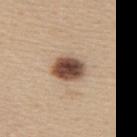Captured during whole-body skin photography for melanoma surveillance; the lesion was not biopsied. Automated image analysis of the tile measured a footprint of about 9.5 mm², a shape eccentricity near 0.65, and a shape-asymmetry score of about 0.15 (0 = symmetric). A close-up tile cropped from a whole-body skin photograph, about 15 mm across. A female subject aged around 45. Located on the upper back.